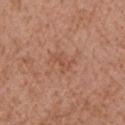Part of a total-body skin-imaging series; this lesion was reviewed on a skin check and was not flagged for biopsy. An algorithmic analysis of the crop reported a mean CIELAB color near L≈52 a*≈23 b*≈31, roughly 6 lightness units darker than nearby skin, and a normalized lesion–skin contrast near 5. It also reported a within-lesion color-variation index near 0.5/10. The recorded lesion diameter is about 3 mm. From the right upper arm. The patient is a female in their mid-70s. Cropped from a whole-body photographic skin survey; the tile spans about 15 mm. The tile uses white-light illumination.